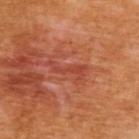The lesion was photographed on a routine skin check and not biopsied; there is no pathology result. The tile uses cross-polarized illumination. The patient is a female aged 53–57. The lesion is on the upper back. A roughly 15 mm field-of-view crop from a total-body skin photograph. Measured at roughly 4.5 mm in maximum diameter.